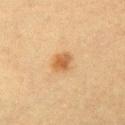follow-up = catalogued during a skin exam; not biopsied
subject = female, roughly 55 years of age
acquisition = ~15 mm tile from a whole-body skin photo
TBP lesion metrics = an average lesion color of about L≈48 a*≈18 b*≈35 (CIELAB), about 9 CIELAB-L* units darker than the surrounding skin, and a normalized border contrast of about 8; lesion-presence confidence of about 100/100
tile lighting = cross-polarized
lesion size = about 2.5 mm
body site = the arm